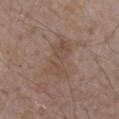Assessment: Recorded during total-body skin imaging; not selected for excision or biopsy. Clinical summary: A lesion tile, about 15 mm wide, cut from a 3D total-body photograph. A male patient, in their mid- to late 70s. About 5 mm across. Automated image analysis of the tile measured a lesion area of about 10 mm² and an eccentricity of roughly 0.9. The software also gave a border-irregularity rating of about 5/10, a color-variation rating of about 2/10, and a peripheral color-asymmetry measure near 0.5. On the arm. This is a white-light tile.